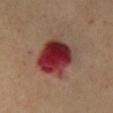Imaged during a routine full-body skin examination; the lesion was not biopsied and no histopathology is available. Measured at roughly 7.5 mm in maximum diameter. The subject is a male approximately 70 years of age. Automated image analysis of the tile measured an area of roughly 23 mm², an outline eccentricity of about 0.75 (0 = round, 1 = elongated), and two-axis asymmetry of about 0.2. The software also gave a mean CIELAB color near L≈34 a*≈29 b*≈23, about 16 CIELAB-L* units darker than the surrounding skin, and a normalized lesion–skin contrast near 14. And it measured an automated nevus-likeness rating near 0 out of 100 and a lesion-detection confidence of about 100/100. Cropped from a whole-body photographic skin survey; the tile spans about 15 mm. Located on the mid back.